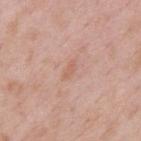Clinical impression:
This lesion was catalogued during total-body skin photography and was not selected for biopsy.
Context:
A 15 mm close-up tile from a total-body photography series done for melanoma screening. The tile uses white-light illumination. Automated tile analysis of the lesion measured a border-irregularity index near 2/10 and internal color variation of about 0.5 on a 0–10 scale. A male subject aged 53–57. On the back.